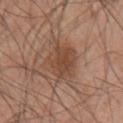Impression:
No biopsy was performed on this lesion — it was imaged during a full skin examination and was not determined to be concerning.
Context:
Cropped from a total-body skin-imaging series; the visible field is about 15 mm. The tile uses white-light illumination. From the left upper arm. A male subject in their mid- to late 40s. An algorithmic analysis of the crop reported a lesion area of about 15 mm², a shape eccentricity near 0.6, and a shape-asymmetry score of about 0.25 (0 = symmetric).